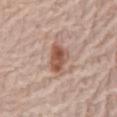workup: imaged on a skin check; not biopsied